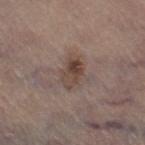| feature | finding |
|---|---|
| biopsy status | imaged on a skin check; not biopsied |
| image source | total-body-photography crop, ~15 mm field of view |
| lesion diameter | about 3.5 mm |
| lighting | white-light illumination |
| site | the right thigh |
| subject | female, in their mid- to late 60s |